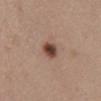The lesion was tiled from a total-body skin photograph and was not biopsied. The lesion-visualizer software estimated an area of roughly 4.5 mm² and an eccentricity of roughly 0.6. And it measured a classifier nevus-likeness of about 100/100 and lesion-presence confidence of about 100/100. A female subject, roughly 55 years of age. A lesion tile, about 15 mm wide, cut from a 3D total-body photograph. Located on the abdomen.